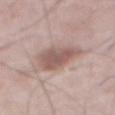Imaged during a routine full-body skin examination; the lesion was not biopsied and no histopathology is available. Cropped from a whole-body photographic skin survey; the tile spans about 15 mm. From the abdomen. A male patient, aged approximately 75.